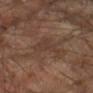Q: Was this lesion biopsied?
A: catalogued during a skin exam; not biopsied
Q: What did automated image analysis measure?
A: a lesion area of about 6.5 mm², a shape eccentricity near 0.8, and a shape-asymmetry score of about 0.6 (0 = symmetric); a classifier nevus-likeness of about 0/100 and a lesion-detection confidence of about 95/100
Q: What is the lesion's diameter?
A: ~4.5 mm (longest diameter)
Q: Where on the body is the lesion?
A: the right forearm
Q: What kind of image is this?
A: ~15 mm crop, total-body skin-cancer survey
Q: Illumination type?
A: cross-polarized
Q: What are the patient's age and sex?
A: male, about 65 years old